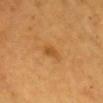Q: Who is the patient?
A: male, aged approximately 60
Q: What did automated image analysis measure?
A: a lesion area of about 3 mm² and a shape eccentricity near 0.85; a lesion color around L≈51 a*≈23 b*≈46 in CIELAB, roughly 8 lightness units darker than nearby skin, and a lesion-to-skin contrast of about 6 (normalized; higher = more distinct); a within-lesion color-variation index near 1/10 and a peripheral color-asymmetry measure near 0.5; a nevus-likeness score of about 25/100
Q: What is the lesion's diameter?
A: about 2.5 mm
Q: What is the imaging modality?
A: 15 mm crop, total-body photography
Q: Illumination type?
A: cross-polarized illumination
Q: Where on the body is the lesion?
A: the chest